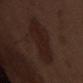Recorded during total-body skin imaging; not selected for excision or biopsy.
The lesion is on the abdomen.
The total-body-photography lesion software estimated a lesion area of about 20 mm², a shape eccentricity near 0.85, and a symmetry-axis asymmetry near 0.2. The analysis additionally found a border-irregularity rating of about 3/10, a color-variation rating of about 2.5/10, and a peripheral color-asymmetry measure near 1. The analysis additionally found a classifier nevus-likeness of about 30/100 and a lesion-detection confidence of about 100/100.
A roughly 15 mm field-of-view crop from a total-body skin photograph.
Longest diameter approximately 7 mm.
A male subject aged 68–72.